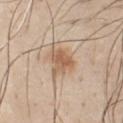This lesion was catalogued during total-body skin photography and was not selected for biopsy.
Automated tile analysis of the lesion measured an area of roughly 7 mm² and a shape eccentricity near 0.1. It also reported a lesion color around L≈59 a*≈18 b*≈32 in CIELAB and a normalized border contrast of about 7.5. The software also gave radial color variation of about 1. The software also gave a detector confidence of about 100 out of 100 that the crop contains a lesion.
A 15 mm crop from a total-body photograph taken for skin-cancer surveillance.
The patient is a male aged 43–47.
The lesion is located on the chest.